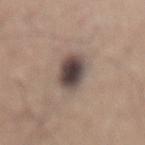The lesion was tiled from a total-body skin photograph and was not biopsied. The lesion is located on the lower back. A male patient aged 33 to 37. A close-up tile cropped from a whole-body skin photograph, about 15 mm across. An algorithmic analysis of the crop reported a color-variation rating of about 7/10. The analysis additionally found a classifier nevus-likeness of about 75/100 and a detector confidence of about 100 out of 100 that the crop contains a lesion. The lesion's longest dimension is about 4.5 mm. The tile uses white-light illumination.